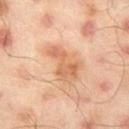Captured during whole-body skin photography for melanoma surveillance; the lesion was not biopsied. The total-body-photography lesion software estimated internal color variation of about 4.5 on a 0–10 scale. The software also gave a classifier nevus-likeness of about 10/100 and a lesion-detection confidence of about 100/100. Captured under cross-polarized illumination. About 5 mm across. On the left thigh. The subject is a male aged 43–47. This image is a 15 mm lesion crop taken from a total-body photograph.